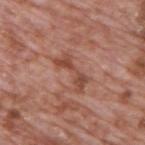The lesion was tiled from a total-body skin photograph and was not biopsied.
A male patient, aged approximately 70.
On the upper back.
About 5 mm across.
A 15 mm crop from a total-body photograph taken for skin-cancer surveillance.